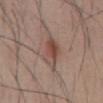Notes:
– biopsy status: no biopsy performed (imaged during a skin exam)
– image: 15 mm crop, total-body photography
– automated lesion analysis: an area of roughly 8.5 mm² and a symmetry-axis asymmetry near 0.25; an average lesion color of about L≈48 a*≈19 b*≈24 (CIELAB) and a lesion-to-skin contrast of about 7 (normalized; higher = more distinct)
– tile lighting: white-light illumination
– subject: male, about 50 years old
– anatomic site: the abdomen
– lesion size: ~4.5 mm (longest diameter)